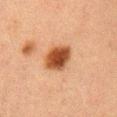Assessment:
Recorded during total-body skin imaging; not selected for excision or biopsy.
Context:
Located on the right upper arm. A male subject aged 48–52. A 15 mm crop from a total-body photograph taken for skin-cancer surveillance. Imaged with cross-polarized lighting. The lesion's longest dimension is about 4 mm. Automated tile analysis of the lesion measured an average lesion color of about L≈40 a*≈22 b*≈32 (CIELAB), a lesion–skin lightness drop of about 14, and a normalized border contrast of about 11.5.